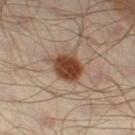The lesion was photographed on a routine skin check and not biopsied; there is no pathology result.
On the leg.
Imaged with cross-polarized lighting.
A roughly 15 mm field-of-view crop from a total-body skin photograph.
The recorded lesion diameter is about 3.5 mm.
The patient is a male aged 63 to 67.